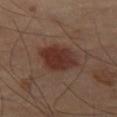Clinical impression: Part of a total-body skin-imaging series; this lesion was reviewed on a skin check and was not flagged for biopsy. Image and clinical context: A region of skin cropped from a whole-body photographic capture, roughly 15 mm wide. The lesion is on the leg. The tile uses cross-polarized illumination. The recorded lesion diameter is about 5 mm.